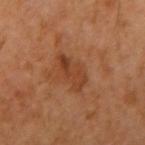| field | value |
|---|---|
| notes | no biopsy performed (imaged during a skin exam) |
| tile lighting | cross-polarized illumination |
| image source | ~15 mm crop, total-body skin-cancer survey |
| anatomic site | the right upper arm |
| subject | in their mid- to late 60s |